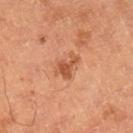No biopsy was performed on this lesion — it was imaged during a full skin examination and was not determined to be concerning.
The lesion-visualizer software estimated a footprint of about 5.5 mm², a shape eccentricity near 0.75, and a shape-asymmetry score of about 0.5 (0 = symmetric). The software also gave an average lesion color of about L≈54 a*≈27 b*≈37 (CIELAB), roughly 10 lightness units darker than nearby skin, and a lesion-to-skin contrast of about 7 (normalized; higher = more distinct). The software also gave a lesion-detection confidence of about 100/100.
About 3 mm across.
This is a cross-polarized tile.
The patient is a male roughly 60 years of age.
The lesion is located on the right lower leg.
A 15 mm close-up tile from a total-body photography series done for melanoma screening.